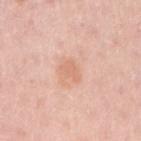Case summary:
* follow-up · catalogued during a skin exam; not biopsied
* size · about 2.5 mm
* acquisition · ~15 mm crop, total-body skin-cancer survey
* patient · female, aged around 30
* anatomic site · the right upper arm